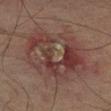| feature | finding |
|---|---|
| biopsy status | imaged on a skin check; not biopsied |
| lesion size | ≈8.5 mm |
| body site | the right lower leg |
| tile lighting | cross-polarized |
| patient | male, in their mid- to late 70s |
| image | ~15 mm crop, total-body skin-cancer survey |